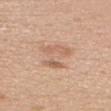Impression: Recorded during total-body skin imaging; not selected for excision or biopsy. Image and clinical context: This image is a 15 mm lesion crop taken from a total-body photograph. The recorded lesion diameter is about 4.5 mm. On the front of the torso. A male patient about 40 years old. The tile uses white-light illumination.